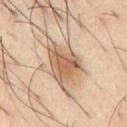{"biopsy_status": "not biopsied; imaged during a skin examination", "site": "chest", "automated_metrics": {"area_mm2_approx": 16.0, "eccentricity": 0.65, "shape_asymmetry": 0.4, "nevus_likeness_0_100": 75, "lesion_detection_confidence_0_100": 100}, "patient": {"sex": "male", "age_approx": 40}, "image": {"source": "total-body photography crop", "field_of_view_mm": 15}}